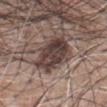Q: Was this lesion biopsied?
A: catalogued during a skin exam; not biopsied
Q: Where on the body is the lesion?
A: the front of the torso
Q: Patient demographics?
A: male, aged 58–62
Q: How large is the lesion?
A: ≈5.5 mm
Q: What lighting was used for the tile?
A: white-light
Q: How was this image acquired?
A: total-body-photography crop, ~15 mm field of view